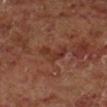No biopsy was performed on this lesion — it was imaged during a full skin examination and was not determined to be concerning.
Approximately 3.5 mm at its widest.
The lesion is on the right lower leg.
This is a cross-polarized tile.
A male patient, roughly 60 years of age.
A close-up tile cropped from a whole-body skin photograph, about 15 mm across.
Automated tile analysis of the lesion measured an area of roughly 5.5 mm², an eccentricity of roughly 0.75, and a symmetry-axis asymmetry near 0.65.